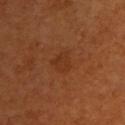<case>
<biopsy_status>not biopsied; imaged during a skin examination</biopsy_status>
<site>upper back</site>
<lighting>cross-polarized</lighting>
<patient>
  <sex>male</sex>
  <age_approx>60</age_approx>
</patient>
<lesion_size>
  <long_diameter_mm_approx>3.0</long_diameter_mm_approx>
</lesion_size>
<image>
  <source>total-body photography crop</source>
  <field_of_view_mm>15</field_of_view_mm>
</image>
</case>